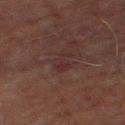Captured during whole-body skin photography for melanoma surveillance; the lesion was not biopsied. Measured at roughly 3 mm in maximum diameter. The patient is a male roughly 70 years of age. A lesion tile, about 15 mm wide, cut from a 3D total-body photograph. The lesion is located on the leg.